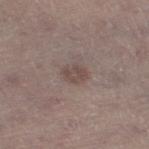Findings:
• biopsy status: total-body-photography surveillance lesion; no biopsy
• image: ~15 mm crop, total-body skin-cancer survey
• patient: male, aged around 65
• TBP lesion metrics: a border-irregularity rating of about 2/10, internal color variation of about 2 on a 0–10 scale, and a peripheral color-asymmetry measure near 0.5; an automated nevus-likeness rating near 15 out of 100 and a detector confidence of about 100 out of 100 that the crop contains a lesion
• anatomic site: the left thigh